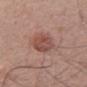  biopsy_status: not biopsied; imaged during a skin examination
  patient:
    sex: male
    age_approx: 60
  image:
    source: total-body photography crop
    field_of_view_mm: 15
  lesion_size:
    long_diameter_mm_approx: 5.0
  site: mid back
  lighting: white-light